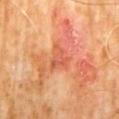This is a cross-polarized tile.
A lesion tile, about 15 mm wide, cut from a 3D total-body photograph.
Longest diameter approximately 3 mm.
The lesion is on the abdomen.
A male patient aged 58 to 62.
Biopsy histopathology demonstrated a squamous cell carcinoma in situ, classified as a malignant skin lesion.